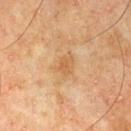Notes:
- biopsy status: no biopsy performed (imaged during a skin exam)
- image-analysis metrics: a footprint of about 4 mm² and an outline eccentricity of about 0.65 (0 = round, 1 = elongated); a lesion color around L≈52 a*≈18 b*≈36 in CIELAB, roughly 7 lightness units darker than nearby skin, and a lesion-to-skin contrast of about 5.5 (normalized; higher = more distinct); border irregularity of about 2 on a 0–10 scale and internal color variation of about 2 on a 0–10 scale
- anatomic site: the front of the torso
- image source: total-body-photography crop, ~15 mm field of view
- diameter: about 2.5 mm
- subject: male, about 65 years old
- tile lighting: cross-polarized illumination